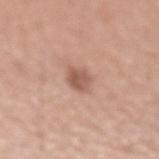Assessment: Captured during whole-body skin photography for melanoma surveillance; the lesion was not biopsied. Image and clinical context: A 15 mm close-up tile from a total-body photography series done for melanoma screening. Captured under white-light illumination. The recorded lesion diameter is about 3 mm. The patient is a male in their 50s. Located on the left forearm.